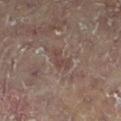| feature | finding |
|---|---|
| follow-up | total-body-photography surveillance lesion; no biopsy |
| lesion size | ≈2.5 mm |
| patient | female, aged 78–82 |
| automated metrics | internal color variation of about 0 on a 0–10 scale and radial color variation of about 0; a nevus-likeness score of about 0/100 and lesion-presence confidence of about 90/100 |
| lighting | cross-polarized |
| site | the right leg |
| image | ~15 mm tile from a whole-body skin photo |